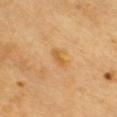The lesion was tiled from a total-body skin photograph and was not biopsied.
About 3 mm across.
A lesion tile, about 15 mm wide, cut from a 3D total-body photograph.
A male subject aged 58–62.
Located on the chest.